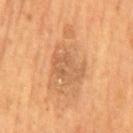workup — total-body-photography surveillance lesion; no biopsy
subject — male, aged 53 to 57
automated lesion analysis — an average lesion color of about L≈55 a*≈20 b*≈36 (CIELAB), about 7 CIELAB-L* units darker than the surrounding skin, and a normalized border contrast of about 5; a border-irregularity index near 4/10, a within-lesion color-variation index near 3/10, and a peripheral color-asymmetry measure near 1; a classifier nevus-likeness of about 0/100 and a lesion-detection confidence of about 100/100
image source — total-body-photography crop, ~15 mm field of view
body site — the mid back
lesion diameter — about 4.5 mm